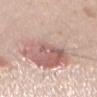Captured during whole-body skin photography for melanoma surveillance; the lesion was not biopsied. The patient is a male in their mid- to late 20s. This image is a 15 mm lesion crop taken from a total-body photograph. Automated tile analysis of the lesion measured a lesion area of about 46 mm², an outline eccentricity of about 0.8 (0 = round, 1 = elongated), and a shape-asymmetry score of about 0.3 (0 = symmetric). It also reported a lesion color around L≈65 a*≈18 b*≈24 in CIELAB, roughly 8 lightness units darker than nearby skin, and a lesion-to-skin contrast of about 5 (normalized; higher = more distinct). Measured at roughly 12 mm in maximum diameter. On the mid back.